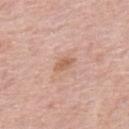<tbp_lesion>
  <biopsy_status>not biopsied; imaged during a skin examination</biopsy_status>
  <lesion_size>
    <long_diameter_mm_approx>2.5</long_diameter_mm_approx>
  </lesion_size>
  <patient>
    <sex>male</sex>
    <age_approx>75</age_approx>
  </patient>
  <lighting>white-light</lighting>
  <image>
    <source>total-body photography crop</source>
    <field_of_view_mm>15</field_of_view_mm>
  </image>
  <site>back</site>
</tbp_lesion>